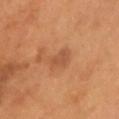Imaged during a routine full-body skin examination; the lesion was not biopsied and no histopathology is available.
A male subject roughly 55 years of age.
The lesion is on the head or neck.
The recorded lesion diameter is about 3.5 mm.
Cropped from a total-body skin-imaging series; the visible field is about 15 mm.